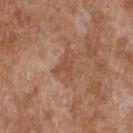No biopsy was performed on this lesion — it was imaged during a full skin examination and was not determined to be concerning. A 15 mm crop from a total-body photograph taken for skin-cancer surveillance. Located on the upper back. The patient is a male aged 43 to 47.